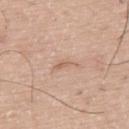workup = total-body-photography surveillance lesion; no biopsy | subject = male, about 75 years old | lesion size = ≈3 mm | image = ~15 mm tile from a whole-body skin photo | lighting = white-light | anatomic site = the back.